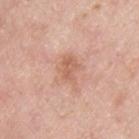| feature | finding |
|---|---|
| workup | total-body-photography surveillance lesion; no biopsy |
| body site | the upper back |
| image source | total-body-photography crop, ~15 mm field of view |
| size | ≈3.5 mm |
| subject | male, about 45 years old |
| lighting | white-light illumination |